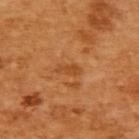Measured at roughly 2.5 mm in maximum diameter. A 15 mm close-up extracted from a 3D total-body photography capture. The lesion-visualizer software estimated an area of roughly 2.5 mm² and an outline eccentricity of about 0.9 (0 = round, 1 = elongated). The analysis additionally found an automated nevus-likeness rating near 0 out of 100 and lesion-presence confidence of about 100/100. On the upper back. The patient is a female about 55 years old. Captured under cross-polarized illumination.